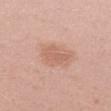| feature | finding |
|---|---|
| biopsy status | imaged on a skin check; not biopsied |
| illumination | white-light illumination |
| automated lesion analysis | an average lesion color of about L≈61 a*≈22 b*≈30 (CIELAB), a lesion–skin lightness drop of about 7, and a normalized lesion–skin contrast near 5; a detector confidence of about 100 out of 100 that the crop contains a lesion |
| size | ~3.5 mm (longest diameter) |
| anatomic site | the right upper arm |
| acquisition | total-body-photography crop, ~15 mm field of view |
| subject | female, roughly 30 years of age |